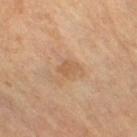Imaged during a routine full-body skin examination; the lesion was not biopsied and no histopathology is available.
The lesion's longest dimension is about 2.5 mm.
The lesion is located on the right thigh.
Imaged with cross-polarized lighting.
A female patient, aged approximately 70.
A 15 mm crop from a total-body photograph taken for skin-cancer surveillance.